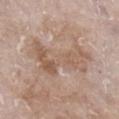follow-up = catalogued during a skin exam; not biopsied | automated lesion analysis = a footprint of about 17 mm² and two-axis asymmetry of about 0.55; an average lesion color of about L≈57 a*≈17 b*≈28 (CIELAB), roughly 8 lightness units darker than nearby skin, and a normalized lesion–skin contrast near 6 | acquisition = ~15 mm tile from a whole-body skin photo | lighting = white-light illumination | subject = female, aged around 75 | site = the right lower leg.